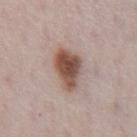This lesion was catalogued during total-body skin photography and was not selected for biopsy. Imaged with white-light lighting. A 15 mm close-up extracted from a 3D total-body photography capture. The lesion is located on the front of the torso. The recorded lesion diameter is about 5.5 mm. A male patient aged 68–72. An algorithmic analysis of the crop reported a footprint of about 13 mm², a shape eccentricity near 0.8, and a shape-asymmetry score of about 0.25 (0 = symmetric). The analysis additionally found an automated nevus-likeness rating near 100 out of 100.